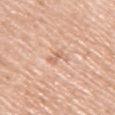Part of a total-body skin-imaging series; this lesion was reviewed on a skin check and was not flagged for biopsy.
Measured at roughly 2.5 mm in maximum diameter.
The lesion is on the right upper arm.
A roughly 15 mm field-of-view crop from a total-body skin photograph.
A male patient aged approximately 70.